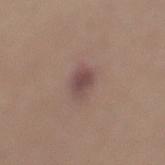Findings:
- biopsy status — total-body-photography surveillance lesion; no biopsy
- size — ≈2.5 mm
- illumination — white-light illumination
- subject — female, roughly 45 years of age
- image — ~15 mm tile from a whole-body skin photo
- anatomic site — the left lower leg
- automated lesion analysis — a lesion area of about 6 mm², a shape eccentricity near 0.2, and a shape-asymmetry score of about 0.2 (0 = symmetric); a classifier nevus-likeness of about 50/100 and a detector confidence of about 100 out of 100 that the crop contains a lesion